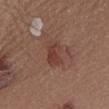<record>
  <biopsy_status>not biopsied; imaged during a skin examination</biopsy_status>
  <image>
    <source>total-body photography crop</source>
    <field_of_view_mm>15</field_of_view_mm>
  </image>
  <site>chest</site>
  <patient>
    <sex>male</sex>
    <age_approx>55</age_approx>
  </patient>
</record>